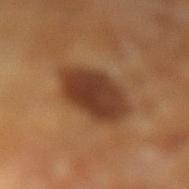Case summary:
• biopsy status: imaged on a skin check; not biopsied
• lighting: cross-polarized
• TBP lesion metrics: a border-irregularity index near 1.5/10, internal color variation of about 3.5 on a 0–10 scale, and peripheral color asymmetry of about 1
• diameter: ≈6 mm
• patient: male, aged approximately 65
• location: the left lower leg
• acquisition: ~15 mm tile from a whole-body skin photo